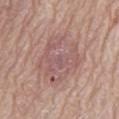- follow-up: catalogued during a skin exam; not biopsied
- anatomic site: the abdomen
- tile lighting: white-light illumination
- automated lesion analysis: a lesion area of about 30 mm², an outline eccentricity of about 0.65 (0 = round, 1 = elongated), and a shape-asymmetry score of about 0.25 (0 = symmetric); a border-irregularity rating of about 3/10, a color-variation rating of about 4.5/10, and peripheral color asymmetry of about 1.5; a classifier nevus-likeness of about 0/100 and a lesion-detection confidence of about 100/100
- acquisition: ~15 mm tile from a whole-body skin photo
- lesion size: ~7.5 mm (longest diameter)
- patient: male, roughly 80 years of age